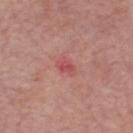Captured during whole-body skin photography for melanoma surveillance; the lesion was not biopsied.